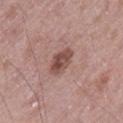Findings:
• biopsy status · no biopsy performed (imaged during a skin exam)
• diameter · ~3.5 mm (longest diameter)
• tile lighting · white-light illumination
• acquisition · total-body-photography crop, ~15 mm field of view
• site · the left thigh
• patient · male, in their 70s
• automated metrics · an area of roughly 6 mm² and an eccentricity of roughly 0.75; a mean CIELAB color near L≈48 a*≈21 b*≈23, a lesion–skin lightness drop of about 12, and a normalized border contrast of about 8.5